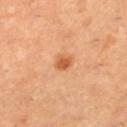Q: Is there a histopathology result?
A: catalogued during a skin exam; not biopsied
Q: What is the imaging modality?
A: ~15 mm tile from a whole-body skin photo
Q: Illumination type?
A: cross-polarized
Q: How large is the lesion?
A: ~2 mm (longest diameter)
Q: What are the patient's age and sex?
A: female, in their 30s
Q: Where on the body is the lesion?
A: the right lower leg